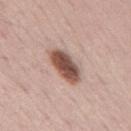Q: Was this lesion biopsied?
A: total-body-photography surveillance lesion; no biopsy
Q: What is the imaging modality?
A: total-body-photography crop, ~15 mm field of view
Q: What is the anatomic site?
A: the mid back
Q: Who is the patient?
A: male, about 70 years old